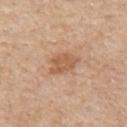Assessment: This lesion was catalogued during total-body skin photography and was not selected for biopsy. Image and clinical context: Longest diameter approximately 3.5 mm. A 15 mm crop from a total-body photograph taken for skin-cancer surveillance. Automated tile analysis of the lesion measured a lesion color around L≈58 a*≈22 b*≈34 in CIELAB, roughly 9 lightness units darker than nearby skin, and a lesion-to-skin contrast of about 6.5 (normalized; higher = more distinct). It also reported border irregularity of about 2.5 on a 0–10 scale, internal color variation of about 2 on a 0–10 scale, and peripheral color asymmetry of about 0.5. It also reported an automated nevus-likeness rating near 10 out of 100 and a lesion-detection confidence of about 100/100. Captured under white-light illumination. A male patient aged approximately 55. Located on the mid back.